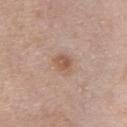Assessment: No biopsy was performed on this lesion — it was imaged during a full skin examination and was not determined to be concerning. Background: The subject is a male aged around 40. This is a white-light tile. The lesion is on the chest. A 15 mm crop from a total-body photograph taken for skin-cancer surveillance.